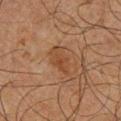- workup — catalogued during a skin exam; not biopsied
- patient — male, in their mid- to late 60s
- anatomic site — the right lower leg
- lesion size — about 4.5 mm
- automated lesion analysis — a lesion area of about 7 mm² and a symmetry-axis asymmetry near 0.3; a border-irregularity rating of about 4/10, a within-lesion color-variation index near 2.5/10, and a peripheral color-asymmetry measure near 0.5; a detector confidence of about 100 out of 100 that the crop contains a lesion
- illumination — cross-polarized illumination
- image — total-body-photography crop, ~15 mm field of view